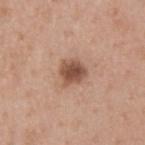Part of a total-body skin-imaging series; this lesion was reviewed on a skin check and was not flagged for biopsy. The subject is a male about 40 years old. A 15 mm close-up tile from a total-body photography series done for melanoma screening. From the upper back. Approximately 3 mm at its widest. This is a white-light tile.